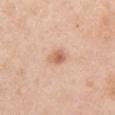follow-up: catalogued during a skin exam; not biopsied | lesion size: ~2.5 mm (longest diameter) | acquisition: 15 mm crop, total-body photography | body site: the arm | patient: female, aged 48 to 52.